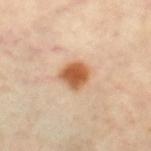{"image": {"source": "total-body photography crop", "field_of_view_mm": 15}, "site": "right lower leg", "automated_metrics": {"nevus_likeness_0_100": 100, "lesion_detection_confidence_0_100": 100}, "lesion_size": {"long_diameter_mm_approx": 3.0}, "patient": {"sex": "female", "age_approx": 65}}